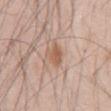<record>
  <biopsy_status>not biopsied; imaged during a skin examination</biopsy_status>
  <automated_metrics>
    <area_mm2_approx>5.0</area_mm2_approx>
    <eccentricity>0.7</eccentricity>
    <shape_asymmetry>0.25</shape_asymmetry>
    <cielab_L>58</cielab_L>
    <cielab_a>20</cielab_a>
    <cielab_b>30</cielab_b>
    <vs_skin_darker_L>9.0</vs_skin_darker_L>
    <vs_skin_contrast_norm>7.0</vs_skin_contrast_norm>
    <border_irregularity_0_10>2.0</border_irregularity_0_10>
    <color_variation_0_10>2.5</color_variation_0_10>
    <nevus_likeness_0_100>85</nevus_likeness_0_100>
    <lesion_detection_confidence_0_100>100</lesion_detection_confidence_0_100>
  </automated_metrics>
  <lighting>white-light</lighting>
  <image>
    <source>total-body photography crop</source>
    <field_of_view_mm>15</field_of_view_mm>
  </image>
  <site>abdomen</site>
  <patient>
    <sex>male</sex>
    <age_approx>45</age_approx>
  </patient>
</record>